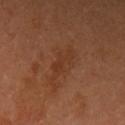– biopsy status · catalogued during a skin exam; not biopsied
– image · ~15 mm tile from a whole-body skin photo
– location · the left upper arm
– subject · female, aged 33–37